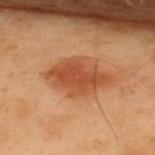Impression: This lesion was catalogued during total-body skin photography and was not selected for biopsy. Context: The patient is a male aged 53 to 57. The recorded lesion diameter is about 5.5 mm. Imaged with cross-polarized lighting. Automated image analysis of the tile measured an area of roughly 16 mm², an eccentricity of roughly 0.75, and a shape-asymmetry score of about 0.2 (0 = symmetric). And it measured a nevus-likeness score of about 100/100. Cropped from a whole-body photographic skin survey; the tile spans about 15 mm. The lesion is located on the upper back.